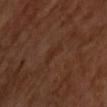Assessment: Recorded during total-body skin imaging; not selected for excision or biopsy.